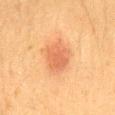• biopsy status: catalogued during a skin exam; not biopsied
• acquisition: 15 mm crop, total-body photography
• illumination: cross-polarized illumination
• site: the back
• subject: male, aged 33 to 37